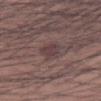biopsy_status: not biopsied; imaged during a skin examination
lesion_size:
  long_diameter_mm_approx: 2.5
image:
  source: total-body photography crop
  field_of_view_mm: 15
site: right upper arm
patient:
  sex: male
  age_approx: 25